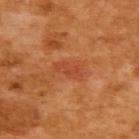follow-up: imaged on a skin check; not biopsied
site: the upper back
illumination: cross-polarized illumination
subject: female, aged 53–57
lesion diameter: ≈3.5 mm
imaging modality: 15 mm crop, total-body photography